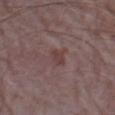Assessment: Imaged during a routine full-body skin examination; the lesion was not biopsied and no histopathology is available. Context: Cropped from a total-body skin-imaging series; the visible field is about 15 mm. On the left forearm. A male patient aged 63–67. The tile uses white-light illumination. The total-body-photography lesion software estimated an area of roughly 3 mm² and a symmetry-axis asymmetry near 0.3. And it measured internal color variation of about 1.5 on a 0–10 scale and radial color variation of about 0.5. The software also gave a classifier nevus-likeness of about 0/100 and a detector confidence of about 100 out of 100 that the crop contains a lesion. Approximately 2.5 mm at its widest.